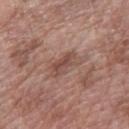biopsy status: no biopsy performed (imaged during a skin exam); patient: female, aged 58–62; diameter: about 4 mm; location: the arm; tile lighting: white-light illumination; image source: ~15 mm tile from a whole-body skin photo.